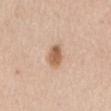The lesion was photographed on a routine skin check and not biopsied; there is no pathology result.
A male subject, roughly 60 years of age.
The recorded lesion diameter is about 3 mm.
The lesion-visualizer software estimated a lesion area of about 5 mm² and a shape eccentricity near 0.7. The software also gave a mean CIELAB color near L≈60 a*≈20 b*≈32, roughly 13 lightness units darker than nearby skin, and a lesion-to-skin contrast of about 8.5 (normalized; higher = more distinct).
From the mid back.
This is a white-light tile.
A 15 mm close-up tile from a total-body photography series done for melanoma screening.